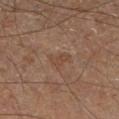Impression: Imaged during a routine full-body skin examination; the lesion was not biopsied and no histopathology is available. Image and clinical context: From the right lower leg. The tile uses cross-polarized illumination. A 15 mm close-up tile from a total-body photography series done for melanoma screening. The patient is a male aged around 65. Measured at roughly 2.5 mm in maximum diameter.